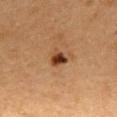No biopsy was performed on this lesion — it was imaged during a full skin examination and was not determined to be concerning. Cropped from a whole-body photographic skin survey; the tile spans about 15 mm. On the mid back. A female subject, aged 38 to 42. Automated tile analysis of the lesion measured an area of roughly 4 mm², a shape eccentricity near 0.6, and a symmetry-axis asymmetry near 0.3. The software also gave a lesion color around L≈34 a*≈21 b*≈30 in CIELAB, roughly 14 lightness units darker than nearby skin, and a normalized lesion–skin contrast near 11.5. The software also gave a nevus-likeness score of about 100/100.